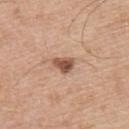Recorded during total-body skin imaging; not selected for excision or biopsy. The lesion is on the upper back. A male subject, approximately 55 years of age. Imaged with white-light lighting. A roughly 15 mm field-of-view crop from a total-body skin photograph.